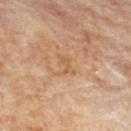{
  "biopsy_status": "not biopsied; imaged during a skin examination",
  "patient": {
    "sex": "male",
    "age_approx": "85+"
  },
  "site": "upper back",
  "lighting": "cross-polarized",
  "lesion_size": {
    "long_diameter_mm_approx": 3.0
  },
  "image": {
    "source": "total-body photography crop",
    "field_of_view_mm": 15
  }
}